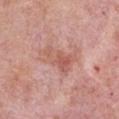The lesion was tiled from a total-body skin photograph and was not biopsied. The tile uses white-light illumination. A male subject, about 75 years old. Longest diameter approximately 4.5 mm. Automated tile analysis of the lesion measured an outline eccentricity of about 0.85 (0 = round, 1 = elongated) and a shape-asymmetry score of about 0.4 (0 = symmetric). It also reported border irregularity of about 5 on a 0–10 scale and a within-lesion color-variation index near 4.5/10. The software also gave a nevus-likeness score of about 0/100 and lesion-presence confidence of about 100/100. A 15 mm crop from a total-body photograph taken for skin-cancer surveillance. The lesion is located on the left upper arm.